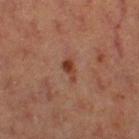Q: What is the imaging modality?
A: 15 mm crop, total-body photography
Q: Lesion location?
A: the left thigh
Q: Patient demographics?
A: female, aged 38 to 42
Q: Automated lesion metrics?
A: a footprint of about 3 mm²; an average lesion color of about L≈32 a*≈21 b*≈26 (CIELAB) and roughly 9 lightness units darker than nearby skin; internal color variation of about 1 on a 0–10 scale
Q: How was the tile lit?
A: cross-polarized